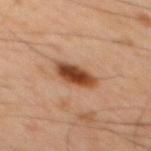{"biopsy_status": "not biopsied; imaged during a skin examination", "patient": {"sex": "male", "age_approx": 45}, "image": {"source": "total-body photography crop", "field_of_view_mm": 15}, "site": "mid back", "lighting": "cross-polarized", "automated_metrics": {"area_mm2_approx": 8.0, "shape_asymmetry": 0.2, "cielab_L": 43, "cielab_a": 23, "cielab_b": 33, "vs_skin_darker_L": 16.0, "vs_skin_contrast_norm": 12.0, "border_irregularity_0_10": 2.0, "color_variation_0_10": 5.5, "nevus_likeness_0_100": 100, "lesion_detection_confidence_0_100": 100}, "lesion_size": {"long_diameter_mm_approx": 4.5}}